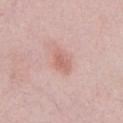Impression:
Imaged during a routine full-body skin examination; the lesion was not biopsied and no histopathology is available.
Context:
This image is a 15 mm lesion crop taken from a total-body photograph. The lesion is on the chest. A male subject, approximately 25 years of age.